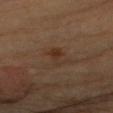notes=catalogued during a skin exam; not biopsied
image source=~15 mm crop, total-body skin-cancer survey
subject=female, aged 68–72
anatomic site=the right forearm
lighting=cross-polarized
diameter=about 3 mm